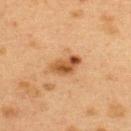Q: How was this image acquired?
A: total-body-photography crop, ~15 mm field of view
Q: What did automated image analysis measure?
A: a lesion area of about 7 mm², a shape eccentricity near 0.8, and a shape-asymmetry score of about 0.3 (0 = symmetric); border irregularity of about 3 on a 0–10 scale, a color-variation rating of about 7/10, and radial color variation of about 3; a nevus-likeness score of about 80/100 and lesion-presence confidence of about 100/100
Q: Lesion location?
A: the upper back
Q: How large is the lesion?
A: about 4 mm
Q: Who is the patient?
A: female, aged 38 to 42